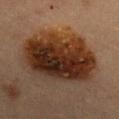| key | value |
|---|---|
| patient | female, aged around 60 |
| anatomic site | the chest |
| tile lighting | cross-polarized illumination |
| image | total-body-photography crop, ~15 mm field of view |